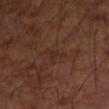The lesion was photographed on a routine skin check and not biopsied; there is no pathology result. A male subject aged around 65. On the left upper arm. Automated image analysis of the tile measured a lesion area of about 2.5 mm², a shape eccentricity near 0.9, and two-axis asymmetry of about 0.5. The software also gave a normalized border contrast of about 4.5. The analysis additionally found a border-irregularity index near 5.5/10 and internal color variation of about 0 on a 0–10 scale. This image is a 15 mm lesion crop taken from a total-body photograph. This is a cross-polarized tile. The lesion's longest dimension is about 2.5 mm.